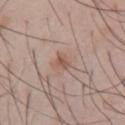Imaged during a routine full-body skin examination; the lesion was not biopsied and no histopathology is available. The total-body-photography lesion software estimated a lesion area of about 3.5 mm², a shape eccentricity near 0.75, and a shape-asymmetry score of about 0.35 (0 = symmetric). The software also gave border irregularity of about 3.5 on a 0–10 scale, internal color variation of about 2.5 on a 0–10 scale, and radial color variation of about 1. The analysis additionally found lesion-presence confidence of about 100/100. A male patient aged 53–57. The lesion is on the chest. Approximately 2.5 mm at its widest. This image is a 15 mm lesion crop taken from a total-body photograph.